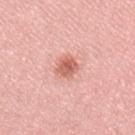{
  "site": "lower back",
  "lighting": "white-light",
  "image": {
    "source": "total-body photography crop",
    "field_of_view_mm": 15
  },
  "patient": {
    "sex": "female",
    "age_approx": 70
  },
  "automated_metrics": {
    "area_mm2_approx": 5.0,
    "eccentricity": 0.45,
    "shape_asymmetry": 0.2,
    "cielab_L": 62,
    "cielab_a": 28,
    "cielab_b": 29,
    "vs_skin_darker_L": 13.0,
    "vs_skin_contrast_norm": 8.0,
    "lesion_detection_confidence_0_100": 100
  }
}